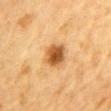notes: no biopsy performed (imaged during a skin exam); body site: the chest; imaging modality: ~15 mm crop, total-body skin-cancer survey; patient: male, approximately 85 years of age or older.